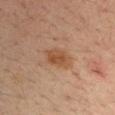{
  "biopsy_status": "not biopsied; imaged during a skin examination",
  "lesion_size": {
    "long_diameter_mm_approx": 3.5
  },
  "automated_metrics": {
    "area_mm2_approx": 7.0,
    "eccentricity": 0.75,
    "border_irregularity_0_10": 2.0,
    "peripheral_color_asymmetry": 1.0,
    "nevus_likeness_0_100": 80,
    "lesion_detection_confidence_0_100": 100
  },
  "patient": {
    "sex": "male",
    "age_approx": 35
  },
  "image": {
    "source": "total-body photography crop",
    "field_of_view_mm": 15
  },
  "lighting": "cross-polarized",
  "site": "left upper arm"
}